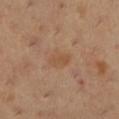• follow-up — no biopsy performed (imaged during a skin exam)
• image — total-body-photography crop, ~15 mm field of view
• site — the left lower leg
• subject — female, aged 28–32